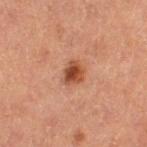Impression: Captured during whole-body skin photography for melanoma surveillance; the lesion was not biopsied. Background: A female subject aged approximately 60. A roughly 15 mm field-of-view crop from a total-body skin photograph. The lesion's longest dimension is about 3 mm. The tile uses cross-polarized illumination. Automated tile analysis of the lesion measured an area of roughly 5 mm² and an eccentricity of roughly 0.55. And it measured a lesion color around L≈39 a*≈22 b*≈29 in CIELAB, a lesion–skin lightness drop of about 11, and a normalized border contrast of about 9.5. The software also gave an automated nevus-likeness rating near 95 out of 100. From the left thigh.